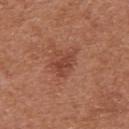Recorded during total-body skin imaging; not selected for excision or biopsy.